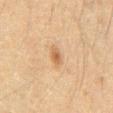Image and clinical context: Imaged with cross-polarized lighting. A male subject aged 58–62. The lesion's longest dimension is about 2.5 mm. Located on the abdomen. The total-body-photography lesion software estimated a border-irregularity rating of about 2.5/10 and internal color variation of about 2.5 on a 0–10 scale. A lesion tile, about 15 mm wide, cut from a 3D total-body photograph.